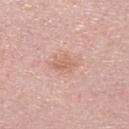Clinical impression: The lesion was photographed on a routine skin check and not biopsied; there is no pathology result. Context: The recorded lesion diameter is about 3 mm. Cropped from a total-body skin-imaging series; the visible field is about 15 mm. A male patient, about 30 years old. Imaged with white-light lighting. Automated image analysis of the tile measured a classifier nevus-likeness of about 5/100.